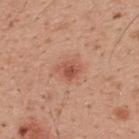biopsy_status: not biopsied; imaged during a skin examination
image:
  source: total-body photography crop
  field_of_view_mm: 15
patient:
  sex: male
  age_approx: 30
lesion_size:
  long_diameter_mm_approx: 3.0
lighting: white-light
site: upper back